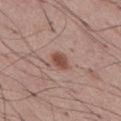Clinical impression: This lesion was catalogued during total-body skin photography and was not selected for biopsy.